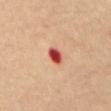biopsy_status: not biopsied; imaged during a skin examination
lesion_size:
  long_diameter_mm_approx: 2.5
lighting: cross-polarized
site: abdomen
patient:
  sex: female
  age_approx: 65
image:
  source: total-body photography crop
  field_of_view_mm: 15